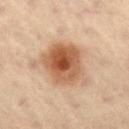| feature | finding |
|---|---|
| notes | no biopsy performed (imaged during a skin exam) |
| subject | male, aged 58 to 62 |
| location | the right thigh |
| size | about 6 mm |
| image source | ~15 mm crop, total-body skin-cancer survey |